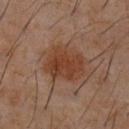Q: Is there a histopathology result?
A: total-body-photography surveillance lesion; no biopsy
Q: What kind of image is this?
A: ~15 mm crop, total-body skin-cancer survey
Q: Patient demographics?
A: male, about 60 years old
Q: What is the anatomic site?
A: the chest